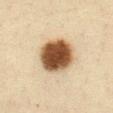Part of a total-body skin-imaging series; this lesion was reviewed on a skin check and was not flagged for biopsy. A 15 mm close-up tile from a total-body photography series done for melanoma screening. Longest diameter approximately 5 mm. A female subject aged around 45. The lesion is on the front of the torso. Automated tile analysis of the lesion measured a lesion color around L≈45 a*≈17 b*≈31 in CIELAB and a normalized border contrast of about 15.5. It also reported a border-irregularity index near 1/10, a color-variation rating of about 5.5/10, and a peripheral color-asymmetry measure near 1.5.